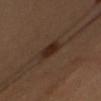<record>
<biopsy_status>not biopsied; imaged during a skin examination</biopsy_status>
<site>right upper arm</site>
<lesion_size>
  <long_diameter_mm_approx>4.0</long_diameter_mm_approx>
</lesion_size>
<image>
  <source>total-body photography crop</source>
  <field_of_view_mm>15</field_of_view_mm>
</image>
<patient>
  <sex>female</sex>
  <age_approx>35</age_approx>
</patient>
</record>